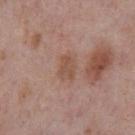follow-up = imaged on a skin check; not biopsied | lighting = white-light illumination | subject = male, roughly 75 years of age | body site = the chest | lesion diameter = about 2.5 mm | imaging modality = ~15 mm crop, total-body skin-cancer survey.